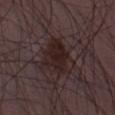The lesion was photographed on a routine skin check and not biopsied; there is no pathology result. Imaged with white-light lighting. About 5 mm across. On the right thigh. A close-up tile cropped from a whole-body skin photograph, about 15 mm across. A male patient, approximately 50 years of age.